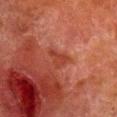The lesion is located on the right lower leg. The recorded lesion diameter is about 3 mm. The tile uses cross-polarized illumination. Cropped from a total-body skin-imaging series; the visible field is about 15 mm. A male patient, about 80 years old.